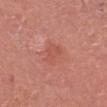Captured during whole-body skin photography for melanoma surveillance; the lesion was not biopsied.
Cropped from a whole-body photographic skin survey; the tile spans about 15 mm.
A male patient in their mid-50s.
Located on the head or neck.